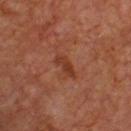<lesion>
  <biopsy_status>not biopsied; imaged during a skin examination</biopsy_status>
  <patient>
    <sex>male</sex>
    <age_approx>65</age_approx>
  </patient>
  <site>chest</site>
  <lighting>cross-polarized</lighting>
  <image>
    <source>total-body photography crop</source>
    <field_of_view_mm>15</field_of_view_mm>
  </image>
  <lesion_size>
    <long_diameter_mm_approx>3.0</long_diameter_mm_approx>
  </lesion_size>
  <automated_metrics>
    <eccentricity>0.9</eccentricity>
    <shape_asymmetry>0.3</shape_asymmetry>
    <cielab_L>35</cielab_L>
    <cielab_a>25</cielab_a>
    <cielab_b>31</cielab_b>
    <vs_skin_darker_L>7.0</vs_skin_darker_L>
    <vs_skin_contrast_norm>7.0</vs_skin_contrast_norm>
    <border_irregularity_0_10>3.0</border_irregularity_0_10>
    <color_variation_0_10>0.5</color_variation_0_10>
    <peripheral_color_asymmetry>0.0</peripheral_color_asymmetry>
  </automated_metrics>
</lesion>